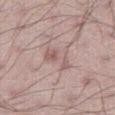Q: What is the anatomic site?
A: the right thigh
Q: What kind of image is this?
A: total-body-photography crop, ~15 mm field of view
Q: Patient demographics?
A: male, aged around 55
Q: What is the lesion's diameter?
A: ~3.5 mm (longest diameter)
Q: What did automated image analysis measure?
A: an area of roughly 8 mm², a shape eccentricity near 0.6, and a shape-asymmetry score of about 0.35 (0 = symmetric); roughly 7 lightness units darker than nearby skin and a lesion-to-skin contrast of about 5 (normalized; higher = more distinct); a classifier nevus-likeness of about 0/100 and lesion-presence confidence of about 100/100